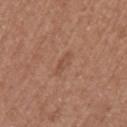This lesion was catalogued during total-body skin photography and was not selected for biopsy. The lesion is on the left upper arm. The subject is a female aged 48–52. A lesion tile, about 15 mm wide, cut from a 3D total-body photograph.